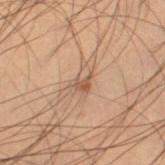Impression:
Recorded during total-body skin imaging; not selected for excision or biopsy.
Background:
A 15 mm close-up extracted from a 3D total-body photography capture. The total-body-photography lesion software estimated border irregularity of about 3.5 on a 0–10 scale, a within-lesion color-variation index near 5/10, and radial color variation of about 1.5. It also reported a nevus-likeness score of about 20/100 and a lesion-detection confidence of about 95/100. A male patient about 55 years old. Captured under cross-polarized illumination. Located on the right thigh.